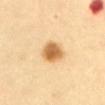Notes:
– notes — catalogued during a skin exam; not biopsied
– lighting — cross-polarized
– anatomic site — the front of the torso
– image-analysis metrics — an eccentricity of roughly 0.45; an automated nevus-likeness rating near 100 out of 100 and lesion-presence confidence of about 100/100
– lesion diameter — ~3.5 mm (longest diameter)
– acquisition — ~15 mm tile from a whole-body skin photo
– subject — female, aged around 60